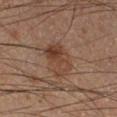follow-up: no biopsy performed (imaged during a skin exam) | illumination: cross-polarized | subject: male, roughly 50 years of age | automated lesion analysis: a border-irregularity index near 4.5/10, internal color variation of about 6 on a 0–10 scale, and radial color variation of about 2.5 | location: the right lower leg | imaging modality: total-body-photography crop, ~15 mm field of view.